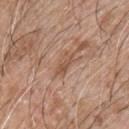Notes:
- follow-up — imaged on a skin check; not biopsied
- subject — male, roughly 65 years of age
- site — the chest
- image source — 15 mm crop, total-body photography